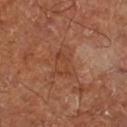Recorded during total-body skin imaging; not selected for excision or biopsy.
This image is a 15 mm lesion crop taken from a total-body photograph.
From the right lower leg.
This is a cross-polarized tile.
The patient is in their mid-60s.
The recorded lesion diameter is about 4.5 mm.